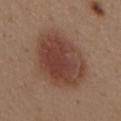The lesion was tiled from a total-body skin photograph and was not biopsied.
From the mid back.
A 15 mm close-up extracted from a 3D total-body photography capture.
Measured at roughly 7.5 mm in maximum diameter.
An algorithmic analysis of the crop reported a lesion area of about 33 mm². The analysis additionally found a detector confidence of about 100 out of 100 that the crop contains a lesion.
A female subject roughly 40 years of age.
Captured under white-light illumination.